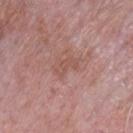Part of a total-body skin-imaging series; this lesion was reviewed on a skin check and was not flagged for biopsy. Cropped from a total-body skin-imaging series; the visible field is about 15 mm. Longest diameter approximately 3 mm. On the chest. An algorithmic analysis of the crop reported a lesion color around L≈53 a*≈22 b*≈25 in CIELAB and a lesion-to-skin contrast of about 5 (normalized; higher = more distinct). Imaged with white-light lighting. A male subject, about 65 years old.